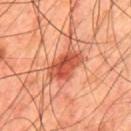Clinical impression:
Part of a total-body skin-imaging series; this lesion was reviewed on a skin check and was not flagged for biopsy.
Image and clinical context:
The recorded lesion diameter is about 4.5 mm. On the back. A male patient, aged approximately 45. A 15 mm close-up extracted from a 3D total-body photography capture. Captured under cross-polarized illumination.